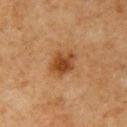Clinical impression: The lesion was photographed on a routine skin check and not biopsied; there is no pathology result. Acquisition and patient details: Cropped from a whole-body photographic skin survey; the tile spans about 15 mm. Located on the arm. The patient is a male in their 60s. Longest diameter approximately 3.5 mm. The total-body-photography lesion software estimated a footprint of about 8 mm² and a shape-asymmetry score of about 0.2 (0 = symmetric). The software also gave an average lesion color of about L≈42 a*≈22 b*≈36 (CIELAB), a lesion–skin lightness drop of about 11, and a normalized lesion–skin contrast near 8.5. It also reported a border-irregularity rating of about 2/10 and a peripheral color-asymmetry measure near 1. The software also gave a classifier nevus-likeness of about 95/100 and a detector confidence of about 100 out of 100 that the crop contains a lesion. Imaged with cross-polarized lighting.